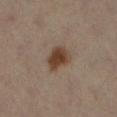workup = imaged on a skin check; not biopsied | anatomic site = the right leg | tile lighting = cross-polarized illumination | imaging modality = ~15 mm tile from a whole-body skin photo | diameter = about 3.5 mm | patient = female, about 40 years old | automated metrics = a lesion color around L≈42 a*≈16 b*≈27 in CIELAB and roughly 11 lightness units darker than nearby skin; a border-irregularity index near 2/10 and peripheral color asymmetry of about 1.